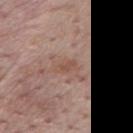- lighting — white-light illumination
- patient — male, aged approximately 75
- imaging modality — 15 mm crop, total-body photography
- diameter — ~3 mm (longest diameter)
- anatomic site — the mid back